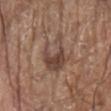{"biopsy_status": "not biopsied; imaged during a skin examination", "site": "abdomen", "automated_metrics": {"cielab_L": 44, "cielab_a": 17, "cielab_b": 24, "vs_skin_darker_L": 9.0, "vs_skin_contrast_norm": 7.5, "border_irregularity_0_10": 7.0, "color_variation_0_10": 3.0, "peripheral_color_asymmetry": 1.0, "nevus_likeness_0_100": 10, "lesion_detection_confidence_0_100": 95}, "patient": {"sex": "male", "age_approx": 80}, "image": {"source": "total-body photography crop", "field_of_view_mm": 15}, "lighting": "white-light", "lesion_size": {"long_diameter_mm_approx": 4.5}}